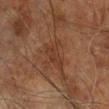Notes:
* biopsy status: imaged on a skin check; not biopsied
* site: the right leg
* subject: male, roughly 60 years of age
* size: about 4 mm
* image: ~15 mm tile from a whole-body skin photo
* lighting: cross-polarized illumination